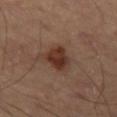<lesion>
<biopsy_status>not biopsied; imaged during a skin examination</biopsy_status>
<image>
  <source>total-body photography crop</source>
  <field_of_view_mm>15</field_of_view_mm>
</image>
<automated_metrics>
  <area_mm2_approx>9.5</area_mm2_approx>
  <eccentricity>0.75</eccentricity>
  <shape_asymmetry>0.25</shape_asymmetry>
  <vs_skin_darker_L>10.0</vs_skin_darker_L>
  <vs_skin_contrast_norm>9.5</vs_skin_contrast_norm>
  <border_irregularity_0_10>3.0</border_irregularity_0_10>
  <peripheral_color_asymmetry>1.5</peripheral_color_asymmetry>
</automated_metrics>
<site>left thigh</site>
</lesion>